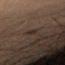Acquisition and patient details:
Automated tile analysis of the lesion measured an area of roughly 2.5 mm², an eccentricity of roughly 0.9, and a shape-asymmetry score of about 0.3 (0 = symmetric). It also reported a mean CIELAB color near L≈21 a*≈10 b*≈15 and a lesion-to-skin contrast of about 6.5 (normalized; higher = more distinct). The analysis additionally found a border-irregularity rating of about 3.5/10 and radial color variation of about 0. A 15 mm crop from a total-body photograph taken for skin-cancer surveillance. The lesion is located on the left thigh. A male subject aged approximately 50. The lesion's longest dimension is about 2.5 mm. This is a cross-polarized tile.